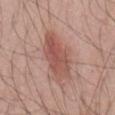tile lighting = white-light illumination; subject = male, aged 43–47; image-analysis metrics = border irregularity of about 3.5 on a 0–10 scale and a peripheral color-asymmetry measure near 1.5; location = the mid back; diameter = ~6.5 mm (longest diameter); image source = ~15 mm tile from a whole-body skin photo.